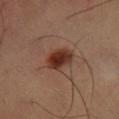The lesion was tiled from a total-body skin photograph and was not biopsied. About 3.5 mm across. Automated image analysis of the tile measured a lesion area of about 7.5 mm², an outline eccentricity of about 0.65 (0 = round, 1 = elongated), and a shape-asymmetry score of about 0.15 (0 = symmetric). The analysis additionally found a mean CIELAB color near L≈33 a*≈22 b*≈27 and roughly 13 lightness units darker than nearby skin. The analysis additionally found a nevus-likeness score of about 100/100 and a lesion-detection confidence of about 100/100. Imaged with cross-polarized lighting. A male patient in their 50s. The lesion is located on the leg. Cropped from a total-body skin-imaging series; the visible field is about 15 mm.